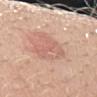Captured during whole-body skin photography for melanoma surveillance; the lesion was not biopsied. A roughly 15 mm field-of-view crop from a total-body skin photograph. The lesion-visualizer software estimated a mean CIELAB color near L≈63 a*≈23 b*≈27 and a lesion-to-skin contrast of about 5 (normalized; higher = more distinct). And it measured a border-irregularity index near 4.5/10, a within-lesion color-variation index near 3/10, and a peripheral color-asymmetry measure near 1. A male patient roughly 30 years of age. Captured under white-light illumination. The lesion is located on the left upper arm. Longest diameter approximately 5 mm.